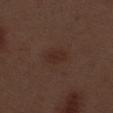follow-up: no biopsy performed (imaged during a skin exam); tile lighting: white-light illumination; imaging modality: total-body-photography crop, ~15 mm field of view; diameter: ~3 mm (longest diameter); subject: male, roughly 70 years of age; site: the left thigh.